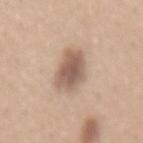No biopsy was performed on this lesion — it was imaged during a full skin examination and was not determined to be concerning. The lesion-visualizer software estimated a footprint of about 12 mm², a shape eccentricity near 0.75, and a shape-asymmetry score of about 0.2 (0 = symmetric). The software also gave an average lesion color of about L≈58 a*≈16 b*≈27 (CIELAB) and a lesion-to-skin contrast of about 8.5 (normalized; higher = more distinct). The software also gave a border-irregularity index near 2/10. The patient is a female roughly 30 years of age. Captured under white-light illumination. Cropped from a whole-body photographic skin survey; the tile spans about 15 mm. The lesion is on the mid back.